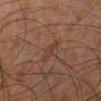<lesion>
  <biopsy_status>not biopsied; imaged during a skin examination</biopsy_status>
  <image>
    <source>total-body photography crop</source>
    <field_of_view_mm>15</field_of_view_mm>
  </image>
  <site>left lower leg</site>
  <patient>
    <sex>male</sex>
    <age_approx>70</age_approx>
  </patient>
</lesion>